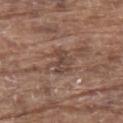Captured during whole-body skin photography for melanoma surveillance; the lesion was not biopsied.
About 3 mm across.
A roughly 15 mm field-of-view crop from a total-body skin photograph.
A male subject, roughly 80 years of age.
Automated image analysis of the tile measured a lesion color around L≈44 a*≈17 b*≈24 in CIELAB, a lesion–skin lightness drop of about 8, and a normalized border contrast of about 6.5.
The lesion is located on the upper back.